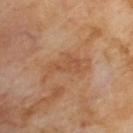Part of a total-body skin-imaging series; this lesion was reviewed on a skin check and was not flagged for biopsy.